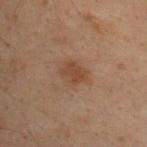Q: Was a biopsy performed?
A: imaged on a skin check; not biopsied
Q: What are the patient's age and sex?
A: male, approximately 30 years of age
Q: What did automated image analysis measure?
A: an area of roughly 5.5 mm² and a shape eccentricity near 0.65; border irregularity of about 2 on a 0–10 scale and a within-lesion color-variation index near 1.5/10
Q: How was the tile lit?
A: cross-polarized illumination
Q: What is the anatomic site?
A: the upper back
Q: How large is the lesion?
A: ≈3 mm
Q: What is the imaging modality?
A: total-body-photography crop, ~15 mm field of view